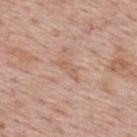workup: catalogued during a skin exam; not biopsied
anatomic site: the upper back
diameter: about 3.5 mm
imaging modality: ~15 mm tile from a whole-body skin photo
subject: male, aged 73–77